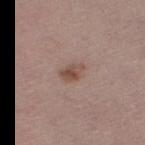Q: What is the imaging modality?
A: ~15 mm crop, total-body skin-cancer survey
Q: Who is the patient?
A: male, aged 58–62
Q: Automated lesion metrics?
A: a footprint of about 4 mm² and two-axis asymmetry of about 0.3; border irregularity of about 3 on a 0–10 scale and a color-variation rating of about 3/10; a nevus-likeness score of about 80/100 and lesion-presence confidence of about 100/100
Q: Lesion size?
A: about 2.5 mm
Q: Lesion location?
A: the leg